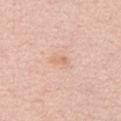{"biopsy_status": "not biopsied; imaged during a skin examination", "automated_metrics": {"cielab_L": 70, "cielab_a": 20, "cielab_b": 32, "vs_skin_darker_L": 6.0, "vs_skin_contrast_norm": 5.0}, "patient": {"sex": "male", "age_approx": 65}, "site": "front of the torso", "image": {"source": "total-body photography crop", "field_of_view_mm": 15}, "lighting": "white-light", "lesion_size": {"long_diameter_mm_approx": 2.5}}